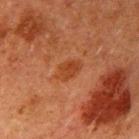Part of a total-body skin-imaging series; this lesion was reviewed on a skin check and was not flagged for biopsy. The lesion-visualizer software estimated a footprint of about 5.5 mm², an eccentricity of roughly 0.8, and a symmetry-axis asymmetry near 0.25. It also reported a nevus-likeness score of about 15/100 and lesion-presence confidence of about 100/100. A male subject approximately 60 years of age. The lesion is located on the right upper arm. A close-up tile cropped from a whole-body skin photograph, about 15 mm across.